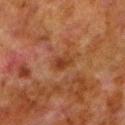<record>
  <site>leg</site>
  <image>
    <source>total-body photography crop</source>
    <field_of_view_mm>15</field_of_view_mm>
  </image>
  <lighting>cross-polarized</lighting>
  <automated_metrics>
    <area_mm2_approx>4.0</area_mm2_approx>
    <eccentricity>0.65</eccentricity>
    <shape_asymmetry>0.25</shape_asymmetry>
    <border_irregularity_0_10>2.5</border_irregularity_0_10>
    <color_variation_0_10>3.5</color_variation_0_10>
    <peripheral_color_asymmetry>1.0</peripheral_color_asymmetry>
    <nevus_likeness_0_100>0</nevus_likeness_0_100>
    <lesion_detection_confidence_0_100>100</lesion_detection_confidence_0_100>
  </automated_metrics>
  <patient>
    <sex>male</sex>
    <age_approx>80</age_approx>
  </patient>
</record>